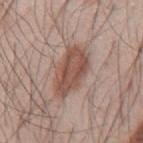Part of a total-body skin-imaging series; this lesion was reviewed on a skin check and was not flagged for biopsy. From the mid back. This image is a 15 mm lesion crop taken from a total-body photograph. The patient is a male aged 43 to 47.